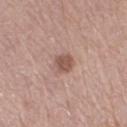{"patient": {"sex": "female", "age_approx": 65}, "site": "left thigh", "image": {"source": "total-body photography crop", "field_of_view_mm": 15}}